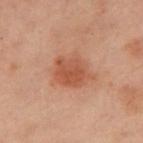Captured during whole-body skin photography for melanoma surveillance; the lesion was not biopsied. A female subject, aged around 55. A region of skin cropped from a whole-body photographic capture, roughly 15 mm wide. Automated tile analysis of the lesion measured an area of roughly 11 mm², an outline eccentricity of about 0.5 (0 = round, 1 = elongated), and two-axis asymmetry of about 0.2. The analysis additionally found border irregularity of about 2 on a 0–10 scale, a within-lesion color-variation index near 3/10, and peripheral color asymmetry of about 1. From the chest.